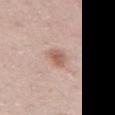Q: Was this lesion biopsied?
A: total-body-photography surveillance lesion; no biopsy
Q: How large is the lesion?
A: about 3 mm
Q: Patient demographics?
A: male, aged 53 to 57
Q: What is the imaging modality?
A: 15 mm crop, total-body photography
Q: Lesion location?
A: the abdomen
Q: What did automated image analysis measure?
A: an area of roughly 5 mm² and two-axis asymmetry of about 0.3; a lesion color around L≈60 a*≈19 b*≈27 in CIELAB, a lesion–skin lightness drop of about 10, and a normalized lesion–skin contrast near 7; a border-irregularity index near 3/10 and radial color variation of about 1
Q: How was the tile lit?
A: white-light